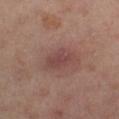No biopsy was performed on this lesion — it was imaged during a full skin examination and was not determined to be concerning. Automated image analysis of the tile measured border irregularity of about 2.5 on a 0–10 scale, a within-lesion color-variation index near 2.5/10, and peripheral color asymmetry of about 1. From the left leg. A lesion tile, about 15 mm wide, cut from a 3D total-body photograph. A female subject aged around 40. The lesion's longest dimension is about 3.5 mm. This is a cross-polarized tile.